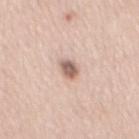Assessment:
Part of a total-body skin-imaging series; this lesion was reviewed on a skin check and was not flagged for biopsy.